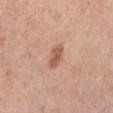The lesion was photographed on a routine skin check and not biopsied; there is no pathology result. A female patient, aged 63–67. A 15 mm close-up tile from a total-body photography series done for melanoma screening. Located on the left lower leg.